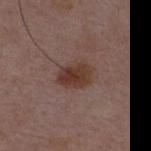The lesion was tiled from a total-body skin photograph and was not biopsied.
A 15 mm close-up tile from a total-body photography series done for melanoma screening.
This is a white-light tile.
The lesion is on the chest.
Automated tile analysis of the lesion measured a lesion color around L≈36 a*≈19 b*≈24 in CIELAB and a lesion–skin lightness drop of about 10. The analysis additionally found a classifier nevus-likeness of about 80/100 and lesion-presence confidence of about 100/100.
A male subject, aged around 50.